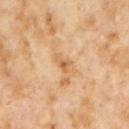This lesion was catalogued during total-body skin photography and was not selected for biopsy. The lesion is located on the chest. Cropped from a whole-body photographic skin survey; the tile spans about 15 mm. A male patient, approximately 60 years of age. Measured at roughly 3 mm in maximum diameter.